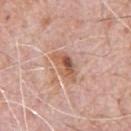Q: Is there a histopathology result?
A: total-body-photography surveillance lesion; no biopsy
Q: What is the imaging modality?
A: total-body-photography crop, ~15 mm field of view
Q: Lesion location?
A: the chest
Q: Who is the patient?
A: male, in their 80s
Q: Automated lesion metrics?
A: a lesion area of about 6.5 mm², a shape eccentricity near 0.85, and a shape-asymmetry score of about 0.3 (0 = symmetric); an automated nevus-likeness rating near 55 out of 100
Q: Illumination type?
A: white-light illumination
Q: What is the lesion's diameter?
A: ~4 mm (longest diameter)